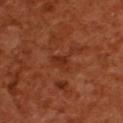Case summary:
- biopsy status — no biopsy performed (imaged during a skin exam)
- diameter — ~2.5 mm (longest diameter)
- image — total-body-photography crop, ~15 mm field of view
- automated lesion analysis — about 7 CIELAB-L* units darker than the surrounding skin and a normalized border contrast of about 6.5
- location — the back
- tile lighting — cross-polarized illumination
- subject — female, aged around 55